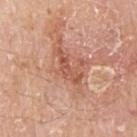biopsy_status: not biopsied; imaged during a skin examination